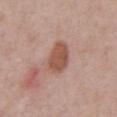Q: Was this lesion biopsied?
A: total-body-photography surveillance lesion; no biopsy
Q: Automated lesion metrics?
A: an area of roughly 9.5 mm², a shape eccentricity near 0.75, and a symmetry-axis asymmetry near 0.2; a mean CIELAB color near L≈53 a*≈22 b*≈27, about 11 CIELAB-L* units darker than the surrounding skin, and a normalized lesion–skin contrast near 8.5
Q: What is the imaging modality?
A: total-body-photography crop, ~15 mm field of view
Q: Patient demographics?
A: male, roughly 70 years of age
Q: Lesion size?
A: ~4 mm (longest diameter)
Q: Illumination type?
A: white-light illumination
Q: Where on the body is the lesion?
A: the chest